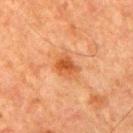This lesion was catalogued during total-body skin photography and was not selected for biopsy. About 3 mm across. Automated tile analysis of the lesion measured an eccentricity of roughly 0.55 and a symmetry-axis asymmetry near 0.25. It also reported a lesion color around L≈44 a*≈25 b*≈36 in CIELAB, a lesion–skin lightness drop of about 9, and a lesion-to-skin contrast of about 7.5 (normalized; higher = more distinct). Captured under cross-polarized illumination. A roughly 15 mm field-of-view crop from a total-body skin photograph. A male subject roughly 80 years of age. The lesion is located on the right upper arm.